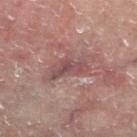| feature | finding |
|---|---|
| automated lesion analysis | a lesion color around L≈42 a*≈19 b*≈17 in CIELAB, about 8 CIELAB-L* units darker than the surrounding skin, and a normalized lesion–skin contrast near 6.5; a classifier nevus-likeness of about 0/100 and a lesion-detection confidence of about 65/100 |
| lesion size | about 4.5 mm |
| anatomic site | the left lower leg |
| patient | male, aged approximately 60 |
| imaging modality | ~15 mm tile from a whole-body skin photo |